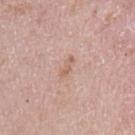notes: catalogued during a skin exam; not biopsied | image: ~15 mm crop, total-body skin-cancer survey | automated lesion analysis: a footprint of about 2.5 mm², an outline eccentricity of about 0.9 (0 = round, 1 = elongated), and a shape-asymmetry score of about 0.3 (0 = symmetric); an average lesion color of about L≈61 a*≈20 b*≈28 (CIELAB), about 8 CIELAB-L* units darker than the surrounding skin, and a normalized lesion–skin contrast near 6; a border-irregularity rating of about 3/10, a within-lesion color-variation index near 0/10, and a peripheral color-asymmetry measure near 0; an automated nevus-likeness rating near 0 out of 100 and a detector confidence of about 100 out of 100 that the crop contains a lesion | patient: female, approximately 50 years of age | diameter: about 2.5 mm | anatomic site: the left upper arm | lighting: white-light illumination.